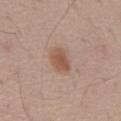Findings:
– biopsy status · imaged on a skin check; not biopsied
– lesion diameter · ~3.5 mm (longest diameter)
– imaging modality · 15 mm crop, total-body photography
– subject · male, in their 60s
– TBP lesion metrics · a footprint of about 6 mm², an eccentricity of roughly 0.75, and two-axis asymmetry of about 0.2; border irregularity of about 2 on a 0–10 scale, internal color variation of about 2.5 on a 0–10 scale, and a peripheral color-asymmetry measure near 1
– anatomic site · the abdomen
– tile lighting · white-light